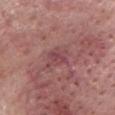The lesion was tiled from a total-body skin photograph and was not biopsied.
The tile uses white-light illumination.
A female subject, in their mid- to late 80s or older.
This image is a 15 mm lesion crop taken from a total-body photograph.
From the head or neck.
An algorithmic analysis of the crop reported a shape eccentricity near 0.35. The analysis additionally found a lesion color around L≈46 a*≈26 b*≈19 in CIELAB, roughly 7 lightness units darker than nearby skin, and a normalized lesion–skin contrast near 5.5. The analysis additionally found border irregularity of about 3 on a 0–10 scale, a within-lesion color-variation index near 4.5/10, and a peripheral color-asymmetry measure near 1.5. And it measured lesion-presence confidence of about 95/100.